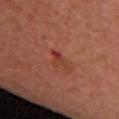Imaged during a routine full-body skin examination; the lesion was not biopsied and no histopathology is available.
Imaged with cross-polarized lighting.
Cropped from a whole-body photographic skin survey; the tile spans about 15 mm.
The lesion is located on the chest.
A female subject, roughly 40 years of age.
About 3 mm across.